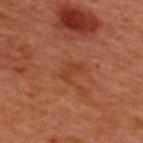Assessment: Captured during whole-body skin photography for melanoma surveillance; the lesion was not biopsied. Clinical summary: The lesion is on the back. A 15 mm crop from a total-body photograph taken for skin-cancer surveillance. Imaged with cross-polarized lighting. Longest diameter approximately 3 mm. A male subject, aged approximately 50. An algorithmic analysis of the crop reported a mean CIELAB color near L≈41 a*≈28 b*≈35, about 6 CIELAB-L* units darker than the surrounding skin, and a normalized border contrast of about 5.5. And it measured border irregularity of about 5 on a 0–10 scale and a color-variation rating of about 0/10.